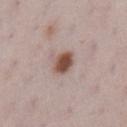Impression:
The lesion was photographed on a routine skin check and not biopsied; there is no pathology result.
Acquisition and patient details:
A close-up tile cropped from a whole-body skin photograph, about 15 mm across. The subject is a female aged around 30. Automated tile analysis of the lesion measured a lesion area of about 6 mm² and a symmetry-axis asymmetry near 0.2. It also reported a lesion color around L≈51 a*≈18 b*≈25 in CIELAB, a lesion–skin lightness drop of about 14, and a normalized border contrast of about 10.5. The recorded lesion diameter is about 3.5 mm. Imaged with white-light lighting. On the left lower leg.